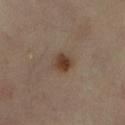biopsy status: total-body-photography surveillance lesion; no biopsy
lighting: cross-polarized illumination
diameter: ≈3 mm
patient: female, roughly 65 years of age
location: the right lower leg
acquisition: total-body-photography crop, ~15 mm field of view
automated metrics: an area of roughly 5 mm² and two-axis asymmetry of about 0.15; border irregularity of about 1.5 on a 0–10 scale and a color-variation rating of about 5/10